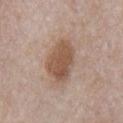  biopsy_status: not biopsied; imaged during a skin examination
  patient:
    sex: male
    age_approx: 55
  site: chest
  automated_metrics:
    vs_skin_darker_L: 11.0
    vs_skin_contrast_norm: 8.5
    nevus_likeness_0_100: 70
    lesion_detection_confidence_0_100: 100
  lesion_size:
    long_diameter_mm_approx: 5.0
  image:
    source: total-body photography crop
    field_of_view_mm: 15